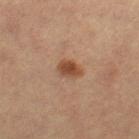  biopsy_status: not biopsied; imaged during a skin examination
  automated_metrics:
    eccentricity: 0.8
    shape_asymmetry: 0.2
    border_irregularity_0_10: 2.0
    color_variation_0_10: 3.5
    peripheral_color_asymmetry: 1.0
    nevus_likeness_0_100: 95
    lesion_detection_confidence_0_100: 100
  lighting: cross-polarized
  site: left thigh
  patient:
    sex: female
    age_approx: 30
  lesion_size:
    long_diameter_mm_approx: 3.0
  image:
    source: total-body photography crop
    field_of_view_mm: 15